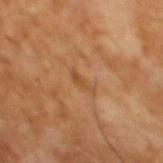The lesion was tiled from a total-body skin photograph and was not biopsied.
The lesion-visualizer software estimated a border-irregularity index near 5.5/10 and internal color variation of about 0 on a 0–10 scale. It also reported an automated nevus-likeness rating near 0 out of 100 and a detector confidence of about 100 out of 100 that the crop contains a lesion.
The patient is a male aged 63 to 67.
A roughly 15 mm field-of-view crop from a total-body skin photograph.
Longest diameter approximately 3 mm.